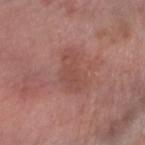This lesion was catalogued during total-body skin photography and was not selected for biopsy.
A female patient roughly 60 years of age.
The tile uses white-light illumination.
A roughly 15 mm field-of-view crop from a total-body skin photograph.
The lesion is on the arm.
The lesion's longest dimension is about 5 mm.